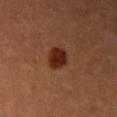This lesion was catalogued during total-body skin photography and was not selected for biopsy. Imaged with cross-polarized lighting. From the right upper arm. The recorded lesion diameter is about 3 mm. The subject is a female roughly 35 years of age. A 15 mm crop from a total-body photograph taken for skin-cancer surveillance.